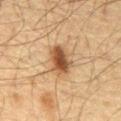Notes:
- follow-up · catalogued during a skin exam; not biopsied
- subject · male, aged 63 to 67
- lighting · cross-polarized
- TBP lesion metrics · about 13 CIELAB-L* units darker than the surrounding skin and a lesion-to-skin contrast of about 10 (normalized; higher = more distinct); a border-irregularity index near 2/10, a color-variation rating of about 5/10, and peripheral color asymmetry of about 1.5; a nevus-likeness score of about 95/100 and a lesion-detection confidence of about 100/100
- site · the abdomen
- lesion diameter · about 4 mm
- image source · ~15 mm tile from a whole-body skin photo